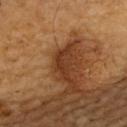biopsy status — total-body-photography surveillance lesion; no biopsy | lesion diameter — ~4 mm (longest diameter) | subject — male, aged around 60 | automated metrics — an area of roughly 4.5 mm², a shape eccentricity near 0.9, and a shape-asymmetry score of about 0.55 (0 = symmetric) | tile lighting — cross-polarized illumination | site — the upper back | image source — 15 mm crop, total-body photography.